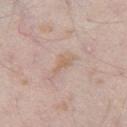workup = imaged on a skin check; not biopsied | image = ~15 mm crop, total-body skin-cancer survey | site = the right thigh | subject = male, approximately 45 years of age | tile lighting = white-light | size = about 3.5 mm.